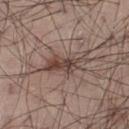A male patient, in their mid- to late 50s. The lesion-visualizer software estimated a border-irregularity rating of about 6.5/10, a color-variation rating of about 6/10, and peripheral color asymmetry of about 2. The recorded lesion diameter is about 5.5 mm. The lesion is on the left thigh. A 15 mm close-up extracted from a 3D total-body photography capture. The tile uses white-light illumination.